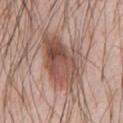Recorded during total-body skin imaging; not selected for excision or biopsy. A 15 mm close-up extracted from a 3D total-body photography capture. The lesion is on the chest. The patient is a male aged approximately 55. The tile uses white-light illumination.